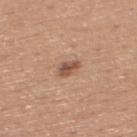Assessment:
Part of a total-body skin-imaging series; this lesion was reviewed on a skin check and was not flagged for biopsy.
Context:
A 15 mm close-up tile from a total-body photography series done for melanoma screening. A male subject approximately 45 years of age. Approximately 2.5 mm at its widest. This is a white-light tile. Located on the upper back.